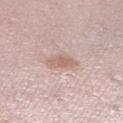The lesion was photographed on a routine skin check and not biopsied; there is no pathology result.
Measured at roughly 3.5 mm in maximum diameter.
A female subject about 45 years old.
The tile uses white-light illumination.
The lesion is located on the right lower leg.
Cropped from a total-body skin-imaging series; the visible field is about 15 mm.